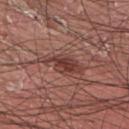Imaged during a routine full-body skin examination; the lesion was not biopsied and no histopathology is available.
About 5.5 mm across.
The lesion is on the upper back.
A male subject in their 40s.
A 15 mm close-up tile from a total-body photography series done for melanoma screening.
The total-body-photography lesion software estimated a lesion area of about 8.5 mm² and an outline eccentricity of about 0.9 (0 = round, 1 = elongated). The software also gave a border-irregularity index near 5/10, a color-variation rating of about 3.5/10, and peripheral color asymmetry of about 1. The software also gave a nevus-likeness score of about 100/100 and lesion-presence confidence of about 100/100.
Imaged with white-light lighting.